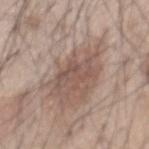Q: Was this lesion biopsied?
A: imaged on a skin check; not biopsied
Q: Patient demographics?
A: male, aged 43 to 47
Q: Where on the body is the lesion?
A: the back
Q: Lesion size?
A: ≈4.5 mm
Q: What kind of image is this?
A: ~15 mm tile from a whole-body skin photo
Q: What lighting was used for the tile?
A: white-light